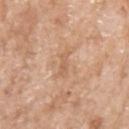Clinical impression: The lesion was photographed on a routine skin check and not biopsied; there is no pathology result.